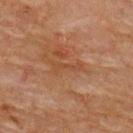Impression: The lesion was tiled from a total-body skin photograph and was not biopsied. Background: The lesion is on the upper back. Automated tile analysis of the lesion measured a lesion area of about 3 mm², an eccentricity of roughly 0.95, and a symmetry-axis asymmetry near 0.45. It also reported a mean CIELAB color near L≈40 a*≈20 b*≈31, about 5 CIELAB-L* units darker than the surrounding skin, and a lesion-to-skin contrast of about 5 (normalized; higher = more distinct). The analysis additionally found a border-irregularity index near 6.5/10 and internal color variation of about 0 on a 0–10 scale. The software also gave a nevus-likeness score of about 0/100 and a detector confidence of about 90 out of 100 that the crop contains a lesion. A female subject in their 80s. This image is a 15 mm lesion crop taken from a total-body photograph.